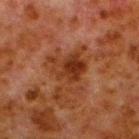A roughly 15 mm field-of-view crop from a total-body skin photograph.
Approximately 5.5 mm at its widest.
The tile uses cross-polarized illumination.
The lesion-visualizer software estimated an area of roughly 13 mm², an eccentricity of roughly 0.6, and two-axis asymmetry of about 0.5. And it measured a classifier nevus-likeness of about 0/100 and a lesion-detection confidence of about 100/100.
Located on the leg.
The subject is a male aged approximately 80.